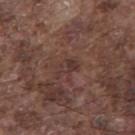A 15 mm close-up tile from a total-body photography series done for melanoma screening. The patient is a male aged 73–77. The lesion is located on the mid back. An algorithmic analysis of the crop reported an area of roughly 4.5 mm² and an eccentricity of roughly 0.8. The analysis additionally found a lesion color around L≈35 a*≈18 b*≈20 in CIELAB, roughly 6 lightness units darker than nearby skin, and a normalized border contrast of about 5.5. And it measured border irregularity of about 3.5 on a 0–10 scale. The lesion's longest dimension is about 3 mm.